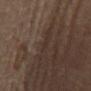Impression: Part of a total-body skin-imaging series; this lesion was reviewed on a skin check and was not flagged for biopsy. Background: On the back. The patient is a female aged 63–67. Automated tile analysis of the lesion measured a footprint of about 1 mm² and an eccentricity of roughly 0.85. The lesion's longest dimension is about 1.5 mm. Imaged with cross-polarized lighting. A region of skin cropped from a whole-body photographic capture, roughly 15 mm wide.